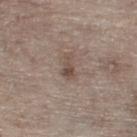Clinical impression: Recorded during total-body skin imaging; not selected for excision or biopsy. Background: A 15 mm close-up extracted from a 3D total-body photography capture. A male subject, roughly 70 years of age. On the right lower leg.